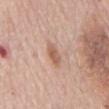{"biopsy_status": "not biopsied; imaged during a skin examination", "lesion_size": {"long_diameter_mm_approx": 3.0}, "lighting": "white-light", "automated_metrics": {"area_mm2_approx": 3.5, "eccentricity": 0.9, "shape_asymmetry": 0.15, "vs_skin_darker_L": 10.0, "vs_skin_contrast_norm": 7.0, "peripheral_color_asymmetry": 0.5, "nevus_likeness_0_100": 55, "lesion_detection_confidence_0_100": 100}, "patient": {"sex": "female", "age_approx": 65}, "image": {"source": "total-body photography crop", "field_of_view_mm": 15}, "site": "chest"}